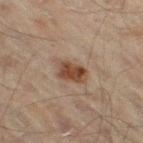workup = imaged on a skin check; not biopsied
automated lesion analysis = a lesion area of about 7 mm², a shape eccentricity near 0.8, and a symmetry-axis asymmetry near 0.25; a lesion color around L≈39 a*≈16 b*≈26 in CIELAB, about 10 CIELAB-L* units darker than the surrounding skin, and a normalized border contrast of about 9.5; border irregularity of about 2.5 on a 0–10 scale, a color-variation rating of about 4.5/10, and peripheral color asymmetry of about 1.5
body site = the leg
image source = total-body-photography crop, ~15 mm field of view
illumination = cross-polarized
patient = male, aged around 45
size = ~4 mm (longest diameter)